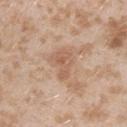Captured during whole-body skin photography for melanoma surveillance; the lesion was not biopsied. A lesion tile, about 15 mm wide, cut from a 3D total-body photograph. Captured under white-light illumination. On the left upper arm. A female patient, about 25 years old.